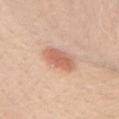Recorded during total-body skin imaging; not selected for excision or biopsy. A roughly 15 mm field-of-view crop from a total-body skin photograph. The patient is a female roughly 25 years of age. On the left upper arm. Automated tile analysis of the lesion measured a lesion area of about 8 mm² and a shape eccentricity near 0.7. The analysis additionally found a lesion-detection confidence of about 100/100. Longest diameter approximately 3.5 mm. The tile uses white-light illumination.